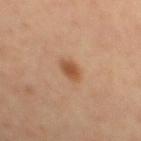This lesion was catalogued during total-body skin photography and was not selected for biopsy. The lesion's longest dimension is about 2.5 mm. This is a cross-polarized tile. An algorithmic analysis of the crop reported a mean CIELAB color near L≈50 a*≈22 b*≈34, a lesion–skin lightness drop of about 10, and a normalized border contrast of about 8. And it measured radial color variation of about 0.5. A male patient aged around 60. A region of skin cropped from a whole-body photographic capture, roughly 15 mm wide. From the mid back.